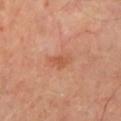Captured during whole-body skin photography for melanoma surveillance; the lesion was not biopsied.